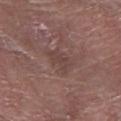Case summary:
– workup · imaged on a skin check; not biopsied
– image-analysis metrics · a mean CIELAB color near L≈42 a*≈18 b*≈20; a nevus-likeness score of about 0/100 and a detector confidence of about 95 out of 100 that the crop contains a lesion
– lesion size · ~3.5 mm (longest diameter)
– location · the left lower leg
– imaging modality · 15 mm crop, total-body photography
– subject · male, in their 80s